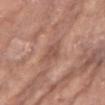This lesion was catalogued during total-body skin photography and was not selected for biopsy. A female patient, approximately 75 years of age. This is a white-light tile. A 15 mm crop from a total-body photograph taken for skin-cancer surveillance. Located on the chest.